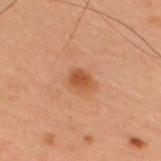{
  "biopsy_status": "not biopsied; imaged during a skin examination",
  "lighting": "cross-polarized",
  "site": "upper back",
  "patient": {
    "sex": "male",
    "age_approx": 55
  },
  "image": {
    "source": "total-body photography crop",
    "field_of_view_mm": 15
  },
  "lesion_size": {
    "long_diameter_mm_approx": 2.5
  },
  "automated_metrics": {
    "area_mm2_approx": 4.0,
    "eccentricity": 0.7,
    "shape_asymmetry": 0.25,
    "cielab_L": 42,
    "cielab_a": 22,
    "cielab_b": 31,
    "vs_skin_darker_L": 8.0,
    "border_irregularity_0_10": 2.0,
    "color_variation_0_10": 2.0,
    "peripheral_color_asymmetry": 1.0
  }
}